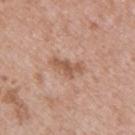Q: Was a biopsy performed?
A: catalogued during a skin exam; not biopsied
Q: What lighting was used for the tile?
A: white-light
Q: How large is the lesion?
A: about 4 mm
Q: Who is the patient?
A: female, aged around 40
Q: How was this image acquired?
A: 15 mm crop, total-body photography
Q: Where on the body is the lesion?
A: the upper back